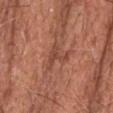Q: Is there a histopathology result?
A: no biopsy performed (imaged during a skin exam)
Q: How was this image acquired?
A: ~15 mm crop, total-body skin-cancer survey
Q: Who is the patient?
A: male, roughly 80 years of age
Q: What is the anatomic site?
A: the head or neck